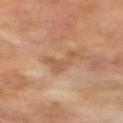Imaged during a routine full-body skin examination; the lesion was not biopsied and no histopathology is available. A male patient aged 68–72. Cropped from a whole-body photographic skin survey; the tile spans about 15 mm. On the right upper arm.